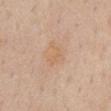{
  "patient": {
    "sex": "male",
    "age_approx": 60
  },
  "lesion_size": {
    "long_diameter_mm_approx": 3.0
  },
  "site": "chest",
  "image": {
    "source": "total-body photography crop",
    "field_of_view_mm": 15
  }
}